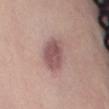Captured during whole-body skin photography for melanoma surveillance; the lesion was not biopsied. A lesion tile, about 15 mm wide, cut from a 3D total-body photograph. Approximately 5 mm at its widest. Captured under white-light illumination. On the back. A female patient roughly 60 years of age.